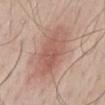A male subject aged approximately 45.
A 15 mm crop from a total-body photograph taken for skin-cancer surveillance.
The lesion-visualizer software estimated a lesion color around L≈57 a*≈22 b*≈26 in CIELAB, about 9 CIELAB-L* units darker than the surrounding skin, and a normalized lesion–skin contrast near 6.5. And it measured an automated nevus-likeness rating near 100 out of 100.
The tile uses white-light illumination.
The lesion is on the mid back.